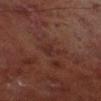Notes:
* follow-up · catalogued during a skin exam; not biopsied
* automated lesion analysis · an automated nevus-likeness rating near 0 out of 100 and a lesion-detection confidence of about 100/100
* acquisition · total-body-photography crop, ~15 mm field of view
* body site · the left lower leg
* patient · male, about 70 years old
* lesion size · about 2.5 mm